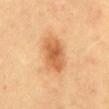follow-up = catalogued during a skin exam; not biopsied | patient = female, aged 58 to 62 | acquisition = total-body-photography crop, ~15 mm field of view | TBP lesion metrics = an area of roughly 13 mm², an eccentricity of roughly 0.75, and two-axis asymmetry of about 0.15; an automated nevus-likeness rating near 95 out of 100 and a detector confidence of about 100 out of 100 that the crop contains a lesion | body site = the front of the torso | tile lighting = cross-polarized | lesion diameter = ~5 mm (longest diameter).